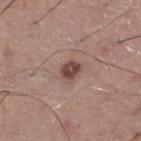Longest diameter approximately 2.5 mm. A male subject, approximately 50 years of age. This is a white-light tile. Located on the left lower leg. A close-up tile cropped from a whole-body skin photograph, about 15 mm across.